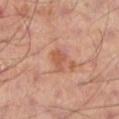Q: Was a biopsy performed?
A: total-body-photography surveillance lesion; no biopsy
Q: What lighting was used for the tile?
A: cross-polarized illumination
Q: Lesion size?
A: about 2.5 mm
Q: What did automated image analysis measure?
A: a shape eccentricity near 0.65 and two-axis asymmetry of about 0.4; a lesion color around L≈51 a*≈24 b*≈30 in CIELAB, about 8 CIELAB-L* units darker than the surrounding skin, and a normalized border contrast of about 6; internal color variation of about 1.5 on a 0–10 scale and peripheral color asymmetry of about 0.5
Q: Patient demographics?
A: male, aged 53–57
Q: How was this image acquired?
A: ~15 mm crop, total-body skin-cancer survey
Q: Where on the body is the lesion?
A: the left lower leg